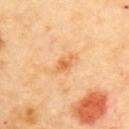| feature | finding |
|---|---|
| notes | total-body-photography surveillance lesion; no biopsy |
| tile lighting | cross-polarized illumination |
| anatomic site | the back |
| lesion size | about 2.5 mm |
| acquisition | ~15 mm crop, total-body skin-cancer survey |
| subject | female, approximately 60 years of age |